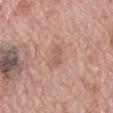Assessment:
Imaged during a routine full-body skin examination; the lesion was not biopsied and no histopathology is available.
Acquisition and patient details:
A male subject in their 70s. The lesion is located on the mid back. Captured under white-light illumination. A 15 mm crop from a total-body photograph taken for skin-cancer surveillance.